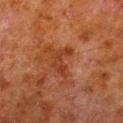Case summary:
– workup · total-body-photography surveillance lesion; no biopsy
– acquisition · 15 mm crop, total-body photography
– site · the right lower leg
– lesion size · about 3.5 mm
– illumination · cross-polarized illumination
– subject · male, roughly 80 years of age